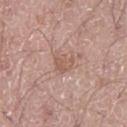| key | value |
|---|---|
| workup | total-body-photography surveillance lesion; no biopsy |
| subject | male, roughly 55 years of age |
| image | total-body-photography crop, ~15 mm field of view |
| body site | the leg |
| lesion diameter | ≈2.5 mm |